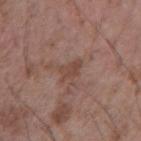Imaged during a routine full-body skin examination; the lesion was not biopsied and no histopathology is available. A male patient, aged 58 to 62. This is a white-light tile. The recorded lesion diameter is about 2.5 mm. On the right forearm. Cropped from a total-body skin-imaging series; the visible field is about 15 mm.